This is a white-light tile, on the head or neck, the patient is a male about 60 years old, approximately 5 mm at its widest, this image is a 15 mm lesion crop taken from a total-body photograph — 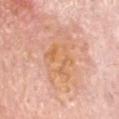{
  "diagnosis": {
    "histopathology": "actinic keratosis",
    "malignancy": "indeterminate",
    "taxonomic_path": [
      "Indeterminate",
      "Indeterminate epidermal proliferations",
      "Solar or actinic keratosis"
    ]
  }
}